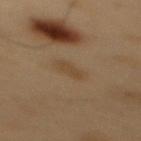The lesion was photographed on a routine skin check and not biopsied; there is no pathology result. An algorithmic analysis of the crop reported a lesion area of about 2.5 mm², an eccentricity of roughly 0.9, and two-axis asymmetry of about 0.35. And it measured border irregularity of about 3.5 on a 0–10 scale, internal color variation of about 0 on a 0–10 scale, and radial color variation of about 0. And it measured an automated nevus-likeness rating near 50 out of 100 and a detector confidence of about 100 out of 100 that the crop contains a lesion. The tile uses cross-polarized illumination. A male patient, about 55 years old. A 15 mm close-up extracted from a 3D total-body photography capture. On the mid back. Approximately 2.5 mm at its widest.